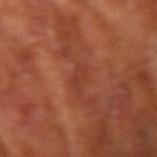| key | value |
|---|---|
| biopsy status | catalogued during a skin exam; not biopsied |
| lighting | cross-polarized |
| imaging modality | ~15 mm crop, total-body skin-cancer survey |
| lesion size | about 3 mm |
| subject | male, aged around 65 |
| site | the right upper arm |